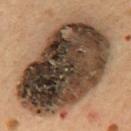| feature | finding |
|---|---|
| workup | no biopsy performed (imaged during a skin exam) |
| image-analysis metrics | a nevus-likeness score of about 0/100 and a detector confidence of about 95 out of 100 that the crop contains a lesion |
| anatomic site | the back |
| tile lighting | cross-polarized |
| image source | 15 mm crop, total-body photography |
| subject | male, in their mid- to late 50s |